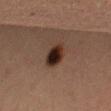No biopsy was performed on this lesion — it was imaged during a full skin examination and was not determined to be concerning. The recorded lesion diameter is about 3.5 mm. This image is a 15 mm lesion crop taken from a total-body photograph. A female patient, aged around 40. Automated image analysis of the tile measured a lesion color around L≈22 a*≈15 b*≈19 in CIELAB, about 13 CIELAB-L* units darker than the surrounding skin, and a lesion-to-skin contrast of about 14 (normalized; higher = more distinct). The analysis additionally found border irregularity of about 2 on a 0–10 scale, a within-lesion color-variation index near 8/10, and a peripheral color-asymmetry measure near 2.5. From the right thigh. The tile uses cross-polarized illumination.